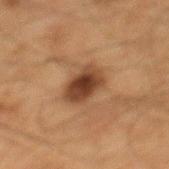{
  "biopsy_status": "not biopsied; imaged during a skin examination",
  "lesion_size": {
    "long_diameter_mm_approx": 4.0
  },
  "lighting": "cross-polarized",
  "site": "mid back",
  "image": {
    "source": "total-body photography crop",
    "field_of_view_mm": 15
  },
  "patient": {
    "sex": "male",
    "age_approx": 65
  },
  "automated_metrics": {
    "shape_asymmetry": 0.15,
    "cielab_L": 34,
    "cielab_a": 18,
    "cielab_b": 28,
    "vs_skin_contrast_norm": 11.0,
    "lesion_detection_confidence_0_100": 100
  }
}